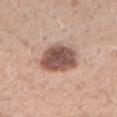Q: Patient demographics?
A: male, aged around 30
Q: What is the imaging modality?
A: total-body-photography crop, ~15 mm field of view
Q: What is the anatomic site?
A: the right forearm